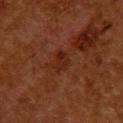Impression: Imaged during a routine full-body skin examination; the lesion was not biopsied and no histopathology is available. Context: The total-body-photography lesion software estimated an area of roughly 4 mm², an outline eccentricity of about 0.75 (0 = round, 1 = elongated), and two-axis asymmetry of about 0.3. And it measured an automated nevus-likeness rating near 0 out of 100 and a detector confidence of about 100 out of 100 that the crop contains a lesion. Imaged with cross-polarized lighting. A 15 mm close-up tile from a total-body photography series done for melanoma screening. The patient is a female aged 48–52. On the upper back. Longest diameter approximately 2.5 mm.